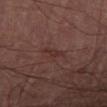lesion diameter: about 3 mm | patient: male, aged approximately 70 | site: the right thigh | acquisition: ~15 mm crop, total-body skin-cancer survey | illumination: cross-polarized.